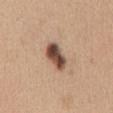Clinical impression: No biopsy was performed on this lesion — it was imaged during a full skin examination and was not determined to be concerning. Image and clinical context: The lesion is located on the chest. A region of skin cropped from a whole-body photographic capture, roughly 15 mm wide. The lesion-visualizer software estimated an eccentricity of roughly 0.85. It also reported internal color variation of about 7 on a 0–10 scale and peripheral color asymmetry of about 2. And it measured a nevus-likeness score of about 95/100 and a detector confidence of about 100 out of 100 that the crop contains a lesion. A female patient, about 30 years old. About 4 mm across.